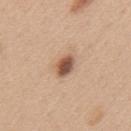The lesion was photographed on a routine skin check and not biopsied; there is no pathology result.
Automated tile analysis of the lesion measured an area of roughly 5 mm², an outline eccentricity of about 0.75 (0 = round, 1 = elongated), and a symmetry-axis asymmetry near 0.2. The software also gave a peripheral color-asymmetry measure near 1.5. And it measured lesion-presence confidence of about 100/100.
The tile uses white-light illumination.
The recorded lesion diameter is about 3 mm.
A female subject, in their 40s.
Located on the mid back.
A lesion tile, about 15 mm wide, cut from a 3D total-body photograph.